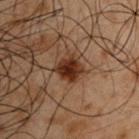The lesion was tiled from a total-body skin photograph and was not biopsied. A 15 mm close-up tile from a total-body photography series done for melanoma screening. On the right upper arm. Approximately 2.5 mm at its widest. Automated tile analysis of the lesion measured a footprint of about 6 mm², an outline eccentricity of about 0.45 (0 = round, 1 = elongated), and a shape-asymmetry score of about 0.25 (0 = symmetric). The software also gave border irregularity of about 2 on a 0–10 scale and radial color variation of about 1. The subject is a male in their 50s. The tile uses cross-polarized illumination.